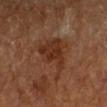{
  "site": "left forearm",
  "lighting": "cross-polarized",
  "patient": {
    "sex": "male",
    "age_approx": "85+"
  },
  "lesion_size": {
    "long_diameter_mm_approx": 6.5
  },
  "automated_metrics": {
    "vs_skin_darker_L": 7.0,
    "vs_skin_contrast_norm": 7.5
  },
  "image": {
    "source": "total-body photography crop",
    "field_of_view_mm": 15
  }
}